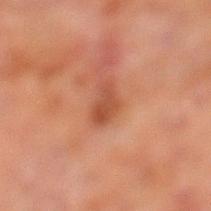The lesion was photographed on a routine skin check and not biopsied; there is no pathology result.
Located on the left lower leg.
The patient is a male roughly 70 years of age.
Imaged with cross-polarized lighting.
Automated tile analysis of the lesion measured a nevus-likeness score of about 5/100.
A roughly 15 mm field-of-view crop from a total-body skin photograph.